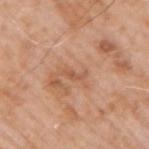Q: Is there a histopathology result?
A: no biopsy performed (imaged during a skin exam)
Q: Illumination type?
A: white-light illumination
Q: How large is the lesion?
A: ≈2.5 mm
Q: Where on the body is the lesion?
A: the right upper arm
Q: What kind of image is this?
A: ~15 mm crop, total-body skin-cancer survey
Q: Who is the patient?
A: male, in their 60s
Q: Automated lesion metrics?
A: a symmetry-axis asymmetry near 0.45; a border-irregularity index near 5/10, internal color variation of about 0 on a 0–10 scale, and radial color variation of about 0